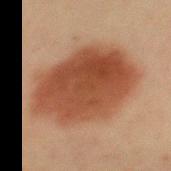Captured during whole-body skin photography for melanoma surveillance; the lesion was not biopsied.
Imaged with cross-polarized lighting.
From the mid back.
A male subject, aged approximately 60.
A 15 mm crop from a total-body photograph taken for skin-cancer surveillance.
An algorithmic analysis of the crop reported internal color variation of about 4.5 on a 0–10 scale and a peripheral color-asymmetry measure near 1.5.